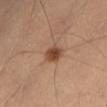A female patient roughly 60 years of age.
A close-up tile cropped from a whole-body skin photograph, about 15 mm across.
On the abdomen.